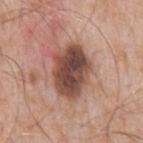biopsy status: no biopsy performed (imaged during a skin exam) | size: about 6 mm | lighting: white-light | body site: the mid back | image source: ~15 mm tile from a whole-body skin photo | automated lesion analysis: an eccentricity of roughly 0.75 and a shape-asymmetry score of about 0.2 (0 = symmetric); a lesion color around L≈46 a*≈21 b*≈25 in CIELAB and roughly 17 lightness units darker than nearby skin | subject: male, about 60 years old.